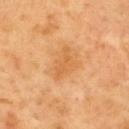The lesion was tiled from a total-body skin photograph and was not biopsied.
The subject is a male aged 48–52.
Located on the mid back.
Automated image analysis of the tile measured an area of roughly 6 mm² and a symmetry-axis asymmetry near 0.5. The software also gave border irregularity of about 5.5 on a 0–10 scale, internal color variation of about 2 on a 0–10 scale, and a peripheral color-asymmetry measure near 0.5. The software also gave an automated nevus-likeness rating near 0 out of 100 and lesion-presence confidence of about 100/100.
A roughly 15 mm field-of-view crop from a total-body skin photograph.